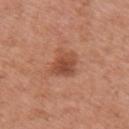Recorded during total-body skin imaging; not selected for excision or biopsy. The recorded lesion diameter is about 3.5 mm. A 15 mm close-up extracted from a 3D total-body photography capture. The lesion is on the right upper arm. A female subject approximately 50 years of age. Automated tile analysis of the lesion measured an area of roughly 8.5 mm², an outline eccentricity of about 0.6 (0 = round, 1 = elongated), and a shape-asymmetry score of about 0.3 (0 = symmetric). The analysis additionally found peripheral color asymmetry of about 1.